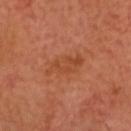follow-up — catalogued during a skin exam; not biopsied | patient — male, in their mid- to late 60s | anatomic site — the head or neck | image source — ~15 mm tile from a whole-body skin photo.